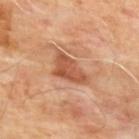Notes:
- site · the upper back
- image source · total-body-photography crop, ~15 mm field of view
- subject · male, aged 63–67
- lesion size · ≈5 mm